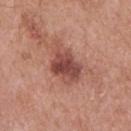Case summary:
– lesion size — about 5.5 mm
– automated metrics — about 12 CIELAB-L* units darker than the surrounding skin and a lesion-to-skin contrast of about 8.5 (normalized; higher = more distinct); a border-irregularity rating of about 3.5/10, a within-lesion color-variation index near 5.5/10, and a peripheral color-asymmetry measure near 2
– site — the upper back
– image source — 15 mm crop, total-body photography
– tile lighting — white-light
– patient — male, approximately 65 years of age